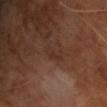notes=no biopsy performed (imaged during a skin exam)
location=the upper back
automated metrics=a border-irregularity index near 6/10 and a color-variation rating of about 0/10
lighting=cross-polarized illumination
imaging modality=~15 mm tile from a whole-body skin photo
size=~3.5 mm (longest diameter)
subject=male, in their 60s